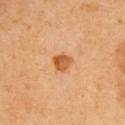Q: Was this lesion biopsied?
A: no biopsy performed (imaged during a skin exam)
Q: What is the anatomic site?
A: the back
Q: How was this image acquired?
A: ~15 mm tile from a whole-body skin photo
Q: What did automated image analysis measure?
A: a lesion color around L≈56 a*≈26 b*≈44 in CIELAB, roughly 12 lightness units darker than nearby skin, and a lesion-to-skin contrast of about 9 (normalized; higher = more distinct)
Q: Who is the patient?
A: female, roughly 65 years of age
Q: Lesion size?
A: about 2.5 mm